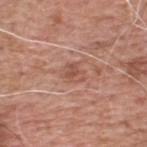Q: Was this lesion biopsied?
A: catalogued during a skin exam; not biopsied
Q: How large is the lesion?
A: ~2.5 mm (longest diameter)
Q: How was the tile lit?
A: white-light
Q: What is the anatomic site?
A: the back
Q: What are the patient's age and sex?
A: male, approximately 60 years of age
Q: What is the imaging modality?
A: ~15 mm crop, total-body skin-cancer survey